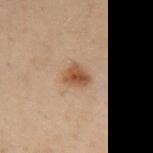Assessment: Imaged during a routine full-body skin examination; the lesion was not biopsied and no histopathology is available. Clinical summary: Imaged with cross-polarized lighting. A male subject, aged around 50. Located on the abdomen. The recorded lesion diameter is about 3 mm. Cropped from a whole-body photographic skin survey; the tile spans about 15 mm. The lesion-visualizer software estimated a lesion area of about 5 mm², an eccentricity of roughly 0.65, and a symmetry-axis asymmetry near 0.25. And it measured a lesion color around L≈38 a*≈16 b*≈26 in CIELAB, about 10 CIELAB-L* units darker than the surrounding skin, and a normalized border contrast of about 9. And it measured a within-lesion color-variation index near 3.5/10.